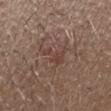The lesion was photographed on a routine skin check and not biopsied; there is no pathology result.
Cropped from a whole-body photographic skin survey; the tile spans about 15 mm.
The lesion's longest dimension is about 4 mm.
Imaged with white-light lighting.
The lesion is located on the head or neck.
The lesion-visualizer software estimated a lesion color around L≈42 a*≈18 b*≈23 in CIELAB, about 6 CIELAB-L* units darker than the surrounding skin, and a lesion-to-skin contrast of about 5.5 (normalized; higher = more distinct). And it measured a nevus-likeness score of about 0/100 and a lesion-detection confidence of about 95/100.
A male subject about 30 years old.